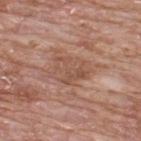Assessment:
Captured during whole-body skin photography for melanoma surveillance; the lesion was not biopsied.
Acquisition and patient details:
Captured under white-light illumination. A close-up tile cropped from a whole-body skin photograph, about 15 mm across. Located on the upper back. A male subject, aged around 65.